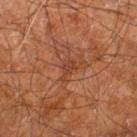{"biopsy_status": "not biopsied; imaged during a skin examination", "patient": {"sex": "male", "age_approx": 60}, "automated_metrics": {"area_mm2_approx": 2.5, "eccentricity": 0.85, "shape_asymmetry": 0.6, "vs_skin_darker_L": 7.0, "vs_skin_contrast_norm": 6.0}, "image": {"source": "total-body photography crop", "field_of_view_mm": 15}, "site": "right leg", "lighting": "cross-polarized", "lesion_size": {"long_diameter_mm_approx": 2.5}}